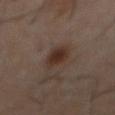Q: Is there a histopathology result?
A: catalogued during a skin exam; not biopsied
Q: How was this image acquired?
A: 15 mm crop, total-body photography
Q: Lesion size?
A: ~3.5 mm (longest diameter)
Q: Lesion location?
A: the abdomen
Q: What are the patient's age and sex?
A: male, approximately 60 years of age